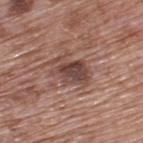Impression: No biopsy was performed on this lesion — it was imaged during a full skin examination and was not determined to be concerning. Acquisition and patient details: On the upper back. A male subject, aged approximately 70. A region of skin cropped from a whole-body photographic capture, roughly 15 mm wide.